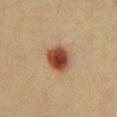No biopsy was performed on this lesion — it was imaged during a full skin examination and was not determined to be concerning. Captured under cross-polarized illumination. A male subject, aged approximately 40. The lesion is on the chest. An algorithmic analysis of the crop reported a mean CIELAB color near L≈41 a*≈21 b*≈31, about 16 CIELAB-L* units darker than the surrounding skin, and a normalized lesion–skin contrast near 12.5. It also reported a border-irregularity index near 1.5/10, internal color variation of about 5.5 on a 0–10 scale, and radial color variation of about 1.5. The analysis additionally found a classifier nevus-likeness of about 100/100. A lesion tile, about 15 mm wide, cut from a 3D total-body photograph. The recorded lesion diameter is about 3.5 mm.